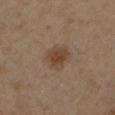| feature | finding |
|---|---|
| biopsy status | no biopsy performed (imaged during a skin exam) |
| subject | male, about 65 years old |
| location | the left lower leg |
| image source | ~15 mm tile from a whole-body skin photo |
| size | ~3.5 mm (longest diameter) |
| TBP lesion metrics | a lesion color around L≈42 a*≈15 b*≈29 in CIELAB, roughly 8 lightness units darker than nearby skin, and a lesion-to-skin contrast of about 7.5 (normalized; higher = more distinct); a nevus-likeness score of about 90/100 and a detector confidence of about 100 out of 100 that the crop contains a lesion |
| illumination | cross-polarized |